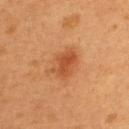biopsy status: no biopsy performed (imaged during a skin exam) | location: the upper back | subject: male, aged around 55 | image: ~15 mm crop, total-body skin-cancer survey | lesion size: ~4 mm (longest diameter) | automated lesion analysis: a footprint of about 9 mm², an outline eccentricity of about 0.7 (0 = round, 1 = elongated), and a shape-asymmetry score of about 0.25 (0 = symmetric); a border-irregularity rating of about 2.5/10 and a color-variation rating of about 3.5/10; a nevus-likeness score of about 90/100 and lesion-presence confidence of about 100/100.